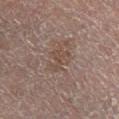Notes:
• biopsy status — total-body-photography surveillance lesion; no biopsy
• diameter — about 3 mm
• image — ~15 mm tile from a whole-body skin photo
• image-analysis metrics — a symmetry-axis asymmetry near 0.55; about 5 CIELAB-L* units darker than the surrounding skin and a normalized lesion–skin contrast near 5; a within-lesion color-variation index near 1/10 and radial color variation of about 0.5
• subject — male, approximately 65 years of age
• illumination — cross-polarized
• location — the right lower leg